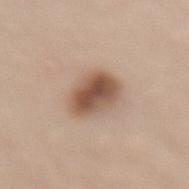The recorded lesion diameter is about 4.5 mm.
A 15 mm close-up extracted from a 3D total-body photography capture.
Imaged with white-light lighting.
The lesion is located on the back.
A female patient, in their 40s.